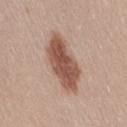| key | value |
|---|---|
| follow-up | no biopsy performed (imaged during a skin exam) |
| lesion diameter | ≈6.5 mm |
| lighting | white-light |
| site | the leg |
| image | total-body-photography crop, ~15 mm field of view |
| TBP lesion metrics | an outline eccentricity of about 0.85 (0 = round, 1 = elongated); peripheral color asymmetry of about 1.5; a nevus-likeness score of about 95/100 and a detector confidence of about 100 out of 100 that the crop contains a lesion |
| patient | female, aged approximately 25 |